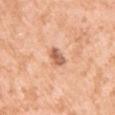follow-up: imaged on a skin check; not biopsied
subject: female, aged around 40
image-analysis metrics: a color-variation rating of about 3.5/10 and a peripheral color-asymmetry measure near 1
acquisition: 15 mm crop, total-body photography
size: about 3 mm
body site: the left upper arm